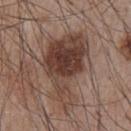Findings:
* follow-up — catalogued during a skin exam; not biopsied